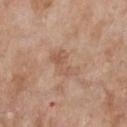| key | value |
|---|---|
| follow-up | total-body-photography surveillance lesion; no biopsy |
| location | the chest |
| lighting | white-light |
| patient | female, aged around 60 |
| image | 15 mm crop, total-body photography |